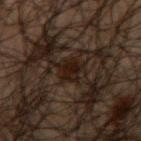Recorded during total-body skin imaging; not selected for excision or biopsy. Measured at roughly 3.5 mm in maximum diameter. Cropped from a total-body skin-imaging series; the visible field is about 15 mm. A male subject approximately 50 years of age. The lesion is on the arm. This is a cross-polarized tile.